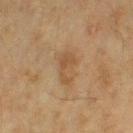workup: imaged on a skin check; not biopsied
automated metrics: a lesion color around L≈44 a*≈16 b*≈31 in CIELAB, roughly 7 lightness units darker than nearby skin, and a lesion-to-skin contrast of about 5.5 (normalized; higher = more distinct); border irregularity of about 6 on a 0–10 scale and radial color variation of about 0; a lesion-detection confidence of about 100/100
location: the leg
illumination: cross-polarized
patient: female, aged around 55
acquisition: total-body-photography crop, ~15 mm field of view